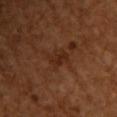No biopsy was performed on this lesion — it was imaged during a full skin examination and was not determined to be concerning.
Approximately 2.5 mm at its widest.
The tile uses cross-polarized illumination.
A female subject in their mid-50s.
Cropped from a total-body skin-imaging series; the visible field is about 15 mm.
The lesion is on the front of the torso.